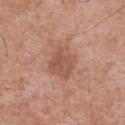This lesion was catalogued during total-body skin photography and was not selected for biopsy. The lesion is located on the chest. Imaged with white-light lighting. The recorded lesion diameter is about 4.5 mm. A 15 mm close-up tile from a total-body photography series done for melanoma screening. The subject is a male aged 68–72.